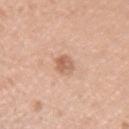Part of a total-body skin-imaging series; this lesion was reviewed on a skin check and was not flagged for biopsy. About 2.5 mm across. Imaged with white-light lighting. From the left upper arm. The patient is a male aged 38 to 42. A region of skin cropped from a whole-body photographic capture, roughly 15 mm wide.